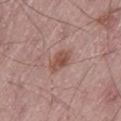Impression:
Recorded during total-body skin imaging; not selected for excision or biopsy.
Background:
The subject is a male in their mid- to late 60s. The recorded lesion diameter is about 3.5 mm. Captured under white-light illumination. A region of skin cropped from a whole-body photographic capture, roughly 15 mm wide. On the left thigh.